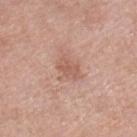workup = total-body-photography surveillance lesion; no biopsy | image = total-body-photography crop, ~15 mm field of view | lesion diameter = ≈2.5 mm | illumination = white-light illumination | anatomic site = the right lower leg | patient = female, approximately 70 years of age.